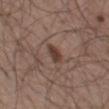{"biopsy_status": "not biopsied; imaged during a skin examination", "patient": {"sex": "male", "age_approx": 75}, "lighting": "white-light", "image": {"source": "total-body photography crop", "field_of_view_mm": 15}, "site": "leg"}